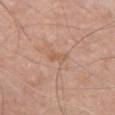On the chest. About 2.5 mm across. This is a white-light tile. A roughly 15 mm field-of-view crop from a total-body skin photograph. A male subject aged 68 to 72. The lesion-visualizer software estimated an area of roughly 3 mm² and a shape eccentricity near 0.9. The analysis additionally found a mean CIELAB color near L≈58 a*≈20 b*≈31, a lesion–skin lightness drop of about 6, and a normalized border contrast of about 5. And it measured a nevus-likeness score of about 0/100 and a lesion-detection confidence of about 100/100.